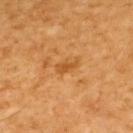workup = total-body-photography surveillance lesion; no biopsy | body site = the upper back | TBP lesion metrics = a lesion–skin lightness drop of about 8 and a normalized lesion–skin contrast near 6.5; a within-lesion color-variation index near 0/10 and radial color variation of about 0; an automated nevus-likeness rating near 0 out of 100 and lesion-presence confidence of about 100/100 | patient = male, about 60 years old | image source = ~15 mm tile from a whole-body skin photo | diameter = ~2.5 mm (longest diameter).